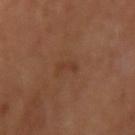Impression:
The lesion was photographed on a routine skin check and not biopsied; there is no pathology result.
Image and clinical context:
A 15 mm crop from a total-body photograph taken for skin-cancer surveillance. The lesion is on the left arm. The subject is a male roughly 50 years of age. The recorded lesion diameter is about 3 mm. Automated tile analysis of the lesion measured an eccentricity of roughly 0.95 and two-axis asymmetry of about 0.45. The analysis additionally found an average lesion color of about L≈36 a*≈21 b*≈29 (CIELAB) and a normalized border contrast of about 5. It also reported lesion-presence confidence of about 100/100.